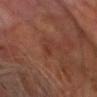follow-up: no biopsy performed (imaged during a skin exam); subject: male, in their 60s; image: total-body-photography crop, ~15 mm field of view; lesion size: about 2.5 mm; lighting: cross-polarized illumination; body site: the head or neck.